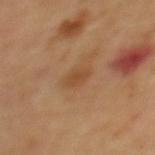Q: Lesion location?
A: the mid back
Q: Automated lesion metrics?
A: an automated nevus-likeness rating near 10 out of 100 and a lesion-detection confidence of about 100/100
Q: What lighting was used for the tile?
A: cross-polarized illumination
Q: What kind of image is this?
A: total-body-photography crop, ~15 mm field of view
Q: Patient demographics?
A: male, aged 63 to 67
Q: How large is the lesion?
A: about 2.5 mm